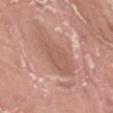Imaged during a routine full-body skin examination; the lesion was not biopsied and no histopathology is available.
The total-body-photography lesion software estimated a mean CIELAB color near L≈57 a*≈21 b*≈27, a lesion–skin lightness drop of about 7, and a lesion-to-skin contrast of about 5 (normalized; higher = more distinct). The software also gave border irregularity of about 2.5 on a 0–10 scale, a color-variation rating of about 1.5/10, and radial color variation of about 0.5. The software also gave a lesion-detection confidence of about 85/100.
A close-up tile cropped from a whole-body skin photograph, about 15 mm across.
The tile uses white-light illumination.
Measured at roughly 5.5 mm in maximum diameter.
From the left thigh.
The subject is a male approximately 40 years of age.